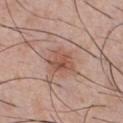notes: total-body-photography surveillance lesion; no biopsy
body site: the chest
patient: male, in their 30s
image: ~15 mm crop, total-body skin-cancer survey